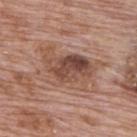workup: catalogued during a skin exam; not biopsied | anatomic site: the upper back | imaging modality: ~15 mm crop, total-body skin-cancer survey | lighting: white-light | patient: male, about 70 years old.